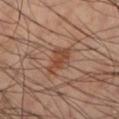The lesion was photographed on a routine skin check and not biopsied; there is no pathology result.
From the left lower leg.
A 15 mm close-up extracted from a 3D total-body photography capture.
The lesion-visualizer software estimated a lesion color around L≈45 a*≈22 b*≈30 in CIELAB, a lesion–skin lightness drop of about 8, and a lesion-to-skin contrast of about 7 (normalized; higher = more distinct). The software also gave lesion-presence confidence of about 100/100.
A male patient, approximately 50 years of age.
The tile uses cross-polarized illumination.